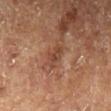Captured during whole-body skin photography for melanoma surveillance; the lesion was not biopsied. This image is a 15 mm lesion crop taken from a total-body photograph. Longest diameter approximately 3 mm. The lesion is located on the left lower leg. A male subject about 75 years old. Captured under cross-polarized illumination.